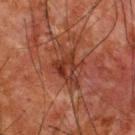The lesion was tiled from a total-body skin photograph and was not biopsied.
This is a cross-polarized tile.
The subject is a male about 65 years old.
The lesion's longest dimension is about 5 mm.
A close-up tile cropped from a whole-body skin photograph, about 15 mm across.
Located on the upper back.